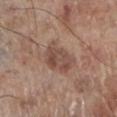Captured during whole-body skin photography for melanoma surveillance; the lesion was not biopsied. A male patient, about 70 years old. A 15 mm crop from a total-body photograph taken for skin-cancer surveillance. Located on the left lower leg.